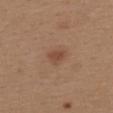Recorded during total-body skin imaging; not selected for excision or biopsy.
Measured at roughly 2.5 mm in maximum diameter.
Automated tile analysis of the lesion measured a lesion color around L≈47 a*≈21 b*≈29 in CIELAB and a lesion–skin lightness drop of about 8. The software also gave a nevus-likeness score of about 55/100 and a lesion-detection confidence of about 100/100.
A 15 mm crop from a total-body photograph taken for skin-cancer surveillance.
This is a white-light tile.
From the upper back.
The subject is a female in their 40s.